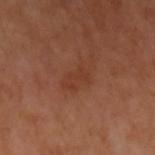Background:
A 15 mm close-up extracted from a 3D total-body photography capture. Located on the left upper arm.